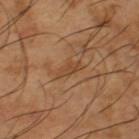notes: total-body-photography surveillance lesion; no biopsy | subject: male, aged around 65 | size: ~3.5 mm (longest diameter) | image: total-body-photography crop, ~15 mm field of view | tile lighting: cross-polarized | body site: the left thigh | TBP lesion metrics: a border-irregularity rating of about 3.5/10 and peripheral color asymmetry of about 0.5.